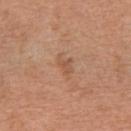A region of skin cropped from a whole-body photographic capture, roughly 15 mm wide. A male patient in their mid- to late 60s. On the abdomen.